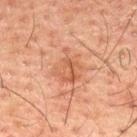Captured during whole-body skin photography for melanoma surveillance; the lesion was not biopsied.
Longest diameter approximately 4 mm.
Imaged with cross-polarized lighting.
A male subject in their 50s.
This image is a 15 mm lesion crop taken from a total-body photograph.
On the upper back.